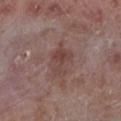subject: male, about 55 years old
imaging modality: ~15 mm tile from a whole-body skin photo
anatomic site: the left lower leg